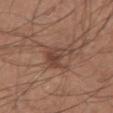Findings:
• workup: catalogued during a skin exam; not biopsied
• site: the left forearm
• image: 15 mm crop, total-body photography
• patient: male, aged 28–32